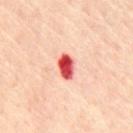Impression: Part of a total-body skin-imaging series; this lesion was reviewed on a skin check and was not flagged for biopsy. Acquisition and patient details: The tile uses cross-polarized illumination. A close-up tile cropped from a whole-body skin photograph, about 15 mm across. A male patient in their mid- to late 60s. From the abdomen. About 3 mm across.